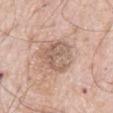biopsy status: imaged on a skin check; not biopsied | image source: 15 mm crop, total-body photography | patient: male, about 80 years old | anatomic site: the abdomen.